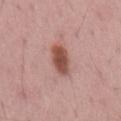Findings:
– biopsy status: catalogued during a skin exam; not biopsied
– illumination: white-light
– site: the mid back
– size: about 4 mm
– patient: male, aged 68–72
– acquisition: total-body-photography crop, ~15 mm field of view